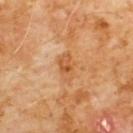| key | value |
|---|---|
| workup | catalogued during a skin exam; not biopsied |
| subject | male, aged 58 to 62 |
| location | the chest |
| illumination | cross-polarized |
| diameter | about 3 mm |
| image source | total-body-photography crop, ~15 mm field of view |